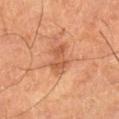biopsy status — imaged on a skin check; not biopsied
illumination — cross-polarized illumination
subject — male, in their 60s
site — the leg
image-analysis metrics — two-axis asymmetry of about 0.35; a detector confidence of about 100 out of 100 that the crop contains a lesion
image source — 15 mm crop, total-body photography
lesion size — about 4 mm